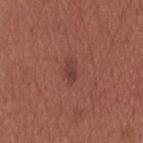Assessment:
Recorded during total-body skin imaging; not selected for excision or biopsy.
Image and clinical context:
The subject is a male in their mid- to late 30s. A 15 mm close-up extracted from a 3D total-body photography capture. From the head or neck. Automated image analysis of the tile measured an eccentricity of roughly 0.85 and two-axis asymmetry of about 0.25.